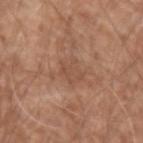Part of a total-body skin-imaging series; this lesion was reviewed on a skin check and was not flagged for biopsy.